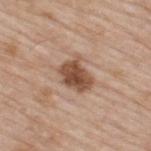A 15 mm crop from a total-body photograph taken for skin-cancer surveillance. Captured under white-light illumination. About 3.5 mm across. The total-body-photography lesion software estimated about 15 CIELAB-L* units darker than the surrounding skin and a normalized lesion–skin contrast near 10. It also reported internal color variation of about 4 on a 0–10 scale and peripheral color asymmetry of about 1.5. From the upper back. A male subject, aged around 65.